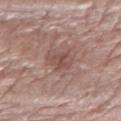biopsy_status: not biopsied; imaged during a skin examination
automated_metrics:
  area_mm2_approx: 5.5
  eccentricity: 0.4
  shape_asymmetry: 0.2
  vs_skin_darker_L: 8.0
  vs_skin_contrast_norm: 6.0
  peripheral_color_asymmetry: 1.0
  lesion_detection_confidence_0_100: 100
lighting: white-light
patient:
  sex: male
  age_approx: 55
image:
  source: total-body photography crop
  field_of_view_mm: 15
lesion_size:
  long_diameter_mm_approx: 3.0
site: right forearm